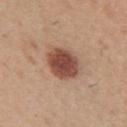Imaged during a routine full-body skin examination; the lesion was not biopsied and no histopathology is available.
The recorded lesion diameter is about 4 mm.
This is a white-light tile.
An algorithmic analysis of the crop reported an area of roughly 13 mm² and a shape-asymmetry score of about 0.15 (0 = symmetric). The analysis additionally found a border-irregularity rating of about 1.5/10, a color-variation rating of about 4/10, and a peripheral color-asymmetry measure near 1. And it measured an automated nevus-likeness rating near 100 out of 100 and a lesion-detection confidence of about 100/100.
A male subject, aged 38–42.
A lesion tile, about 15 mm wide, cut from a 3D total-body photograph.
Located on the arm.